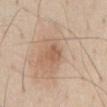tile lighting: white-light illumination | TBP lesion metrics: a footprint of about 3.5 mm² and a shape-asymmetry score of about 0.25 (0 = symmetric); a border-irregularity index near 2.5/10, a color-variation rating of about 1.5/10, and a peripheral color-asymmetry measure near 0.5; a nevus-likeness score of about 25/100 and a detector confidence of about 100 out of 100 that the crop contains a lesion | image: ~15 mm crop, total-body skin-cancer survey | patient: male, aged approximately 60 | location: the chest | size: ≈2 mm.